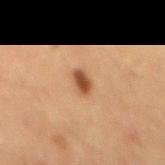biopsy status: imaged on a skin check; not biopsied | lesion size: ≈2.5 mm | tile lighting: cross-polarized | imaging modality: ~15 mm crop, total-body skin-cancer survey | TBP lesion metrics: a footprint of about 3.5 mm² and a shape-asymmetry score of about 0.25 (0 = symmetric); a nevus-likeness score of about 100/100 and a lesion-detection confidence of about 100/100 | patient: male, aged 58–62.